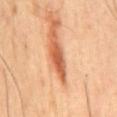Q: Is there a histopathology result?
A: total-body-photography surveillance lesion; no biopsy
Q: Patient demographics?
A: male, roughly 45 years of age
Q: What is the lesion's diameter?
A: ~4 mm (longest diameter)
Q: How was this image acquired?
A: ~15 mm crop, total-body skin-cancer survey
Q: What did automated image analysis measure?
A: an average lesion color of about L≈54 a*≈30 b*≈38 (CIELAB) and roughly 16 lightness units darker than nearby skin; a nevus-likeness score of about 100/100
Q: How was the tile lit?
A: cross-polarized
Q: What is the anatomic site?
A: the mid back